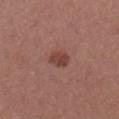Impression:
Imaged during a routine full-body skin examination; the lesion was not biopsied and no histopathology is available.
Background:
A female subject, aged around 20. This image is a 15 mm lesion crop taken from a total-body photograph. Automated tile analysis of the lesion measured a mean CIELAB color near L≈43 a*≈23 b*≈24, about 9 CIELAB-L* units darker than the surrounding skin, and a normalized border contrast of about 7.5. It also reported lesion-presence confidence of about 100/100. The recorded lesion diameter is about 2.5 mm. Located on the left upper arm.